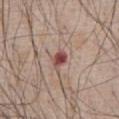Background: A male patient aged 63–67. The lesion is located on the chest. This image is a 15 mm lesion crop taken from a total-body photograph.